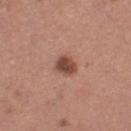No biopsy was performed on this lesion — it was imaged during a full skin examination and was not determined to be concerning.
The recorded lesion diameter is about 3 mm.
A 15 mm close-up extracted from a 3D total-body photography capture.
Located on the left thigh.
The subject is a female in their 30s.